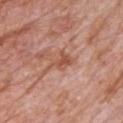Captured during whole-body skin photography for melanoma surveillance; the lesion was not biopsied. A male patient in their 80s. Captured under white-light illumination. A lesion tile, about 15 mm wide, cut from a 3D total-body photograph. Located on the chest.